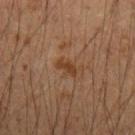{"biopsy_status": "not biopsied; imaged during a skin examination", "image": {"source": "total-body photography crop", "field_of_view_mm": 15}, "automated_metrics": {"border_irregularity_0_10": 3.5, "color_variation_0_10": 1.0, "peripheral_color_asymmetry": 0.5}, "patient": {"sex": "male", "age_approx": 50}, "lighting": "cross-polarized", "lesion_size": {"long_diameter_mm_approx": 2.5}, "site": "left forearm"}